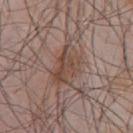Imaged during a routine full-body skin examination; the lesion was not biopsied and no histopathology is available. The tile uses white-light illumination. A male subject, aged approximately 55. This image is a 15 mm lesion crop taken from a total-body photograph. About 4.5 mm across. The lesion is on the chest. An algorithmic analysis of the crop reported a lesion color around L≈46 a*≈17 b*≈24 in CIELAB and a normalized lesion–skin contrast near 7. The analysis additionally found border irregularity of about 4 on a 0–10 scale, internal color variation of about 4 on a 0–10 scale, and radial color variation of about 1.5. The analysis additionally found lesion-presence confidence of about 100/100.